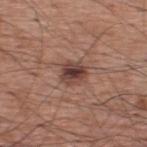Clinical impression: Recorded during total-body skin imaging; not selected for excision or biopsy. Context: Automated image analysis of the tile measured a mean CIELAB color near L≈41 a*≈20 b*≈23 and a lesion-to-skin contrast of about 10 (normalized; higher = more distinct). It also reported border irregularity of about 2.5 on a 0–10 scale, internal color variation of about 6 on a 0–10 scale, and peripheral color asymmetry of about 2. The recorded lesion diameter is about 3 mm. Located on the upper back. A male patient roughly 60 years of age. Cropped from a whole-body photographic skin survey; the tile spans about 15 mm.